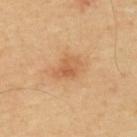A male subject approximately 65 years of age. From the upper back. A 15 mm close-up tile from a total-body photography series done for melanoma screening.